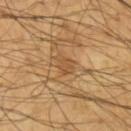Notes:
- notes — imaged on a skin check; not biopsied
- patient — male, aged around 65
- acquisition — ~15 mm tile from a whole-body skin photo
- tile lighting — cross-polarized
- anatomic site — the left forearm
- lesion size — ≈3 mm